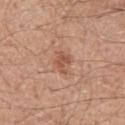{
  "biopsy_status": "not biopsied; imaged during a skin examination",
  "patient": {
    "sex": "male",
    "age_approx": 50
  },
  "site": "chest",
  "automated_metrics": {
    "eccentricity": 0.8,
    "shape_asymmetry": 0.45,
    "border_irregularity_0_10": 4.5,
    "color_variation_0_10": 2.5,
    "peripheral_color_asymmetry": 1.0,
    "lesion_detection_confidence_0_100": 100
  },
  "image": {
    "source": "total-body photography crop",
    "field_of_view_mm": 15
  }
}